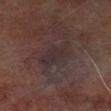Clinical impression: The lesion was photographed on a routine skin check and not biopsied; there is no pathology result. Context: A male patient aged 68 to 72. The tile uses cross-polarized illumination. Located on the right lower leg. A 15 mm close-up extracted from a 3D total-body photography capture. An algorithmic analysis of the crop reported a footprint of about 10 mm², an outline eccentricity of about 0.85 (0 = round, 1 = elongated), and a symmetry-axis asymmetry near 0.35. And it measured an average lesion color of about L≈22 a*≈11 b*≈11 (CIELAB), roughly 4 lightness units darker than nearby skin, and a lesion-to-skin contrast of about 6 (normalized; higher = more distinct). The analysis additionally found a border-irregularity index near 4.5/10 and internal color variation of about 2.5 on a 0–10 scale. About 5 mm across.